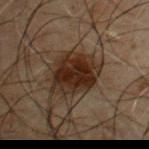Impression:
The lesion was tiled from a total-body skin photograph and was not biopsied.
Background:
Longest diameter approximately 5.5 mm. A roughly 15 mm field-of-view crop from a total-body skin photograph. Captured under cross-polarized illumination. Located on the chest. The subject is a male aged around 50. The total-body-photography lesion software estimated an average lesion color of about L≈19 a*≈13 b*≈20 (CIELAB), about 10 CIELAB-L* units darker than the surrounding skin, and a normalized border contrast of about 12.5. The analysis additionally found an automated nevus-likeness rating near 90 out of 100 and a detector confidence of about 100 out of 100 that the crop contains a lesion.